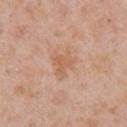  biopsy_status: not biopsied; imaged during a skin examination
  image:
    source: total-body photography crop
    field_of_view_mm: 15
  site: right upper arm
  automated_metrics:
    area_mm2_approx: 7.5
    eccentricity: 0.6
    shape_asymmetry: 0.4
    vs_skin_contrast_norm: 5.5
    color_variation_0_10: 2.5
    peripheral_color_asymmetry: 1.0
    nevus_likeness_0_100: 0
    lesion_detection_confidence_0_100: 100
  lighting: white-light
  patient:
    sex: female
    age_approx: 40
  lesion_size:
    long_diameter_mm_approx: 3.5